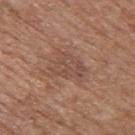This lesion was catalogued during total-body skin photography and was not selected for biopsy. A 15 mm close-up extracted from a 3D total-body photography capture. From the upper back. The patient is a male aged approximately 75.